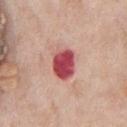{"biopsy_status": "not biopsied; imaged during a skin examination", "patient": {"sex": "male", "age_approx": 75}, "image": {"source": "total-body photography crop", "field_of_view_mm": 15}, "lesion_size": {"long_diameter_mm_approx": 4.0}, "site": "chest", "lighting": "white-light"}